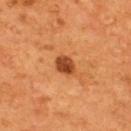Assessment:
The lesion was tiled from a total-body skin photograph and was not biopsied.
Acquisition and patient details:
Cropped from a total-body skin-imaging series; the visible field is about 15 mm. A male patient aged 53 to 57. Longest diameter approximately 2.5 mm. From the back.